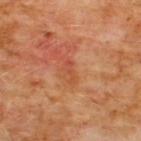| field | value |
|---|---|
| follow-up | catalogued during a skin exam; not biopsied |
| lesion diameter | about 2.5 mm |
| image-analysis metrics | a mean CIELAB color near L≈48 a*≈29 b*≈36 and a lesion–skin lightness drop of about 6; a color-variation rating of about 0/10 and radial color variation of about 0; an automated nevus-likeness rating near 0 out of 100 and a detector confidence of about 100 out of 100 that the crop contains a lesion |
| illumination | cross-polarized |
| image source | ~15 mm crop, total-body skin-cancer survey |
| location | the chest |
| subject | male, aged approximately 60 |